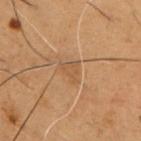  biopsy_status: not biopsied; imaged during a skin examination
  image:
    source: total-body photography crop
    field_of_view_mm: 15
  lighting: cross-polarized
  patient:
    sex: male
    age_approx: 50
  automated_metrics:
    area_mm2_approx: 3.5
    eccentricity: 0.6
    shape_asymmetry: 0.55
    cielab_L: 41
    cielab_a: 16
    cielab_b: 30
    vs_skin_darker_L: 5.0
    vs_skin_contrast_norm: 4.5
    nevus_likeness_0_100: 0
    lesion_detection_confidence_0_100: 95
  site: chest